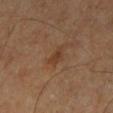Q: Was this lesion biopsied?
A: no biopsy performed (imaged during a skin exam)
Q: Patient demographics?
A: male, approximately 60 years of age
Q: How large is the lesion?
A: ~2.5 mm (longest diameter)
Q: What lighting was used for the tile?
A: cross-polarized illumination
Q: What is the imaging modality?
A: ~15 mm tile from a whole-body skin photo
Q: What is the anatomic site?
A: the right leg
Q: What did automated image analysis measure?
A: a lesion area of about 3 mm² and an outline eccentricity of about 0.8 (0 = round, 1 = elongated); a lesion color around L≈36 a*≈18 b*≈29 in CIELAB, about 6 CIELAB-L* units darker than the surrounding skin, and a normalized border contrast of about 6; an automated nevus-likeness rating near 5 out of 100 and a detector confidence of about 100 out of 100 that the crop contains a lesion